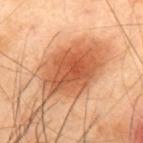| key | value |
|---|---|
| image | ~15 mm crop, total-body skin-cancer survey |
| subject | male, aged 38 to 42 |
| anatomic site | the back |
| tile lighting | cross-polarized illumination |
| automated metrics | a lesion color around L≈58 a*≈29 b*≈40 in CIELAB and a normalized lesion–skin contrast near 8.5 |